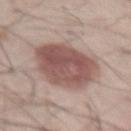This lesion was catalogued during total-body skin photography and was not selected for biopsy.
About 6.5 mm across.
A male subject about 65 years old.
A lesion tile, about 15 mm wide, cut from a 3D total-body photograph.
Imaged with white-light lighting.
On the front of the torso.